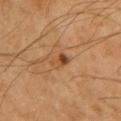  biopsy_status: not biopsied; imaged during a skin examination
  site: left upper arm
  patient:
    sex: male
    age_approx: 70
  image:
    source: total-body photography crop
    field_of_view_mm: 15